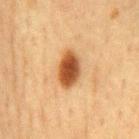Findings:
– notes — total-body-photography surveillance lesion; no biopsy
– anatomic site — the mid back
– image — ~15 mm crop, total-body skin-cancer survey
– subject — male, approximately 75 years of age